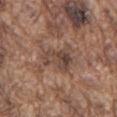workup: catalogued during a skin exam; not biopsied | imaging modality: 15 mm crop, total-body photography | image-analysis metrics: a lesion area of about 9.5 mm², an eccentricity of roughly 0.55, and a symmetry-axis asymmetry near 0.45; a color-variation rating of about 6/10 and a peripheral color-asymmetry measure near 2.5; a lesion-detection confidence of about 90/100 | lighting: white-light | location: the mid back | patient: male, aged 73 to 77.